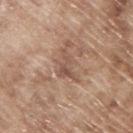{"biopsy_status": "not biopsied; imaged during a skin examination", "lesion_size": {"long_diameter_mm_approx": 4.5}, "automated_metrics": {"area_mm2_approx": 5.5, "eccentricity": 0.85, "shape_asymmetry": 0.6, "color_variation_0_10": 1.5, "peripheral_color_asymmetry": 0.5}, "patient": {"sex": "male", "age_approx": 65}, "site": "back", "image": {"source": "total-body photography crop", "field_of_view_mm": 15}, "lighting": "white-light"}